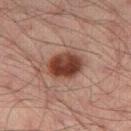Clinical impression:
Captured during whole-body skin photography for melanoma surveillance; the lesion was not biopsied.
Context:
Captured under cross-polarized illumination. A male patient aged 33 to 37. The total-body-photography lesion software estimated roughly 15 lightness units darker than nearby skin and a normalized border contrast of about 12.5. And it measured border irregularity of about 1.5 on a 0–10 scale, a within-lesion color-variation index near 5.5/10, and a peripheral color-asymmetry measure near 2. From the right thigh. A 15 mm close-up extracted from a 3D total-body photography capture.